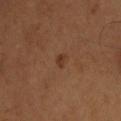Assessment: Part of a total-body skin-imaging series; this lesion was reviewed on a skin check and was not flagged for biopsy. Image and clinical context: This is a cross-polarized tile. The lesion is on the upper back. A close-up tile cropped from a whole-body skin photograph, about 15 mm across. A male subject aged around 65. Automated tile analysis of the lesion measured an area of roughly 1.5 mm², a shape eccentricity near 0.65, and a symmetry-axis asymmetry near 0.35. The analysis additionally found roughly 7 lightness units darker than nearby skin and a normalized lesion–skin contrast near 7.